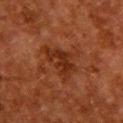notes: catalogued during a skin exam; not biopsied | size: ≈5 mm | lighting: cross-polarized illumination | subject: female, aged 48–52 | body site: the upper back | image: 15 mm crop, total-body photography.